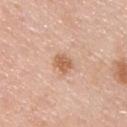biopsy status: catalogued during a skin exam; not biopsied | automated metrics: roughly 11 lightness units darker than nearby skin; a classifier nevus-likeness of about 45/100 and lesion-presence confidence of about 100/100 | lighting: white-light illumination | acquisition: 15 mm crop, total-body photography | patient: male, in their mid- to late 40s | body site: the chest | lesion diameter: about 2.5 mm.